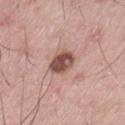The patient is a male aged around 50. Cropped from a total-body skin-imaging series; the visible field is about 15 mm. Imaged with white-light lighting. The lesion is on the left thigh. Automated tile analysis of the lesion measured an eccentricity of roughly 0.65 and a shape-asymmetry score of about 0.15 (0 = symmetric). The analysis additionally found roughly 16 lightness units darker than nearby skin and a normalized lesion–skin contrast near 10.5. The analysis additionally found an automated nevus-likeness rating near 50 out of 100 and a detector confidence of about 100 out of 100 that the crop contains a lesion. Measured at roughly 3.5 mm in maximum diameter.